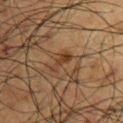Assessment: Part of a total-body skin-imaging series; this lesion was reviewed on a skin check and was not flagged for biopsy. Clinical summary: A male patient, approximately 60 years of age. The lesion is located on the right upper arm. Longest diameter approximately 3 mm. Automated image analysis of the tile measured a border-irregularity rating of about 4/10, a color-variation rating of about 1/10, and peripheral color asymmetry of about 0. A lesion tile, about 15 mm wide, cut from a 3D total-body photograph.